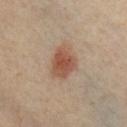biopsy status = total-body-photography surveillance lesion; no biopsy
subject = female, aged 38–42
acquisition = ~15 mm tile from a whole-body skin photo
lighting = cross-polarized illumination
site = the chest